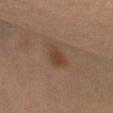A female patient, approximately 30 years of age.
A 15 mm close-up tile from a total-body photography series done for melanoma screening.
From the left thigh.